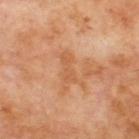- biopsy status: catalogued during a skin exam; not biopsied
- lesion diameter: about 4.5 mm
- tile lighting: cross-polarized illumination
- subject: male, aged approximately 70
- anatomic site: the back
- image source: ~15 mm tile from a whole-body skin photo